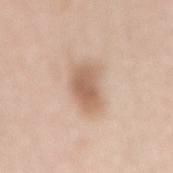The lesion was photographed on a routine skin check and not biopsied; there is no pathology result. A region of skin cropped from a whole-body photographic capture, roughly 15 mm wide. Imaged with white-light lighting. Located on the mid back. A female subject, aged around 70.